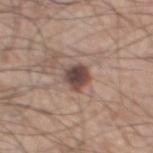The lesion was tiled from a total-body skin photograph and was not biopsied.
This image is a 15 mm lesion crop taken from a total-body photograph.
A male patient, approximately 60 years of age.
Approximately 3 mm at its widest.
Imaged with white-light lighting.
The lesion is located on the left thigh.